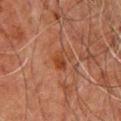No biopsy was performed on this lesion — it was imaged during a full skin examination and was not determined to be concerning. The patient is a male aged approximately 60. The lesion is located on the chest. The total-body-photography lesion software estimated a lesion area of about 4 mm² and a shape eccentricity near 0.75. It also reported a mean CIELAB color near L≈33 a*≈22 b*≈29 and a normalized border contrast of about 7. The software also gave a border-irregularity rating of about 3.5/10 and internal color variation of about 3 on a 0–10 scale. And it measured a classifier nevus-likeness of about 0/100 and lesion-presence confidence of about 100/100. A roughly 15 mm field-of-view crop from a total-body skin photograph. Imaged with cross-polarized lighting. About 3 mm across.